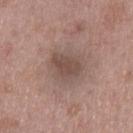Findings:
• workup · total-body-photography surveillance lesion; no biopsy
• lighting · white-light illumination
• lesion diameter · ~4 mm (longest diameter)
• subject · male, roughly 50 years of age
• image-analysis metrics · a shape eccentricity near 0.65 and two-axis asymmetry of about 0.3; a detector confidence of about 100 out of 100 that the crop contains a lesion
• imaging modality · ~15 mm tile from a whole-body skin photo
• site · the left thigh